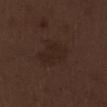| key | value |
|---|---|
| biopsy status | no biopsy performed (imaged during a skin exam) |
| lesion diameter | ~4 mm (longest diameter) |
| patient | male, aged around 70 |
| imaging modality | 15 mm crop, total-body photography |
| image-analysis metrics | a footprint of about 12 mm², an outline eccentricity of about 0.45 (0 = round, 1 = elongated), and a symmetry-axis asymmetry near 0.25; border irregularity of about 2.5 on a 0–10 scale, a color-variation rating of about 2.5/10, and peripheral color asymmetry of about 1 |
| anatomic site | the right lower leg |